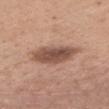{"lesion_size": {"long_diameter_mm_approx": 6.0}, "lighting": "white-light", "patient": {"sex": "female", "age_approx": 40}, "site": "chest", "image": {"source": "total-body photography crop", "field_of_view_mm": 15}}